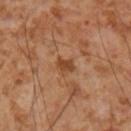Q: Was a biopsy performed?
A: no biopsy performed (imaged during a skin exam)
Q: How was this image acquired?
A: ~15 mm crop, total-body skin-cancer survey
Q: Patient demographics?
A: male, about 55 years old
Q: Where on the body is the lesion?
A: the right upper arm